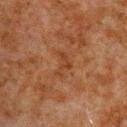Impression:
Part of a total-body skin-imaging series; this lesion was reviewed on a skin check and was not flagged for biopsy.
Context:
A male patient, aged approximately 80. The lesion is located on the right upper arm. A close-up tile cropped from a whole-body skin photograph, about 15 mm across. The lesion's longest dimension is about 3 mm.